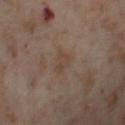Imaged during a routine full-body skin examination; the lesion was not biopsied and no histopathology is available.
Captured under cross-polarized illumination.
A female subject, approximately 55 years of age.
Measured at roughly 2.5 mm in maximum diameter.
On the left thigh.
A 15 mm crop from a total-body photograph taken for skin-cancer surveillance.